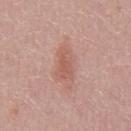Impression: This lesion was catalogued during total-body skin photography and was not selected for biopsy. Context: This is a white-light tile. A male subject, in their mid- to late 40s. Located on the chest. Approximately 4 mm at its widest. A region of skin cropped from a whole-body photographic capture, roughly 15 mm wide.